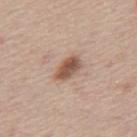biopsy status — imaged on a skin check; not biopsied
image — ~15 mm crop, total-body skin-cancer survey
anatomic site — the mid back
subject — male, in their mid- to late 40s
tile lighting — white-light illumination
automated lesion analysis — an outline eccentricity of about 0.85 (0 = round, 1 = elongated) and two-axis asymmetry of about 0.3; a lesion color around L≈53 a*≈19 b*≈28 in CIELAB, a lesion–skin lightness drop of about 14, and a normalized lesion–skin contrast near 9.5; a classifier nevus-likeness of about 90/100 and a detector confidence of about 100 out of 100 that the crop contains a lesion